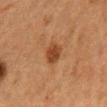An algorithmic analysis of the crop reported an average lesion color of about L≈36 a*≈21 b*≈31 (CIELAB) and a lesion-to-skin contrast of about 8.5 (normalized; higher = more distinct). It also reported an automated nevus-likeness rating near 85 out of 100 and lesion-presence confidence of about 100/100.
Measured at roughly 2.5 mm in maximum diameter.
On the mid back.
A male patient roughly 65 years of age.
This is a cross-polarized tile.
A region of skin cropped from a whole-body photographic capture, roughly 15 mm wide.